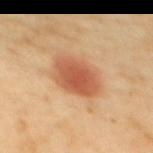- notes — no biopsy performed (imaged during a skin exam)
- size — about 5 mm
- automated lesion analysis — a lesion area of about 14 mm² and a symmetry-axis asymmetry near 0.15; a nevus-likeness score of about 100/100
- body site — the back
- acquisition — ~15 mm tile from a whole-body skin photo
- subject — female, aged 58–62
- illumination — cross-polarized